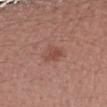Impression: The lesion was tiled from a total-body skin photograph and was not biopsied. Acquisition and patient details: Located on the left forearm. A female patient, in their mid-50s. A roughly 15 mm field-of-view crop from a total-body skin photograph.